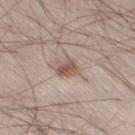Context:
Captured under white-light illumination. The lesion is on the right thigh. A male subject in their mid- to late 20s. A 15 mm close-up extracted from a 3D total-body photography capture. The recorded lesion diameter is about 2.5 mm.